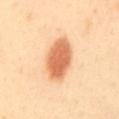biopsy_status: not biopsied; imaged during a skin examination
lighting: cross-polarized
image:
  source: total-body photography crop
  field_of_view_mm: 15
lesion_size:
  long_diameter_mm_approx: 6.0
automated_metrics:
  cielab_L: 69
  cielab_a: 27
  cielab_b: 42
  vs_skin_darker_L: 17.0
  vs_skin_contrast_norm: 9.5
  border_irregularity_0_10: 1.5
  peripheral_color_asymmetry: 1.0
  nevus_likeness_0_100: 100
  lesion_detection_confidence_0_100: 100
patient:
  sex: female
  age_approx: 40
site: back